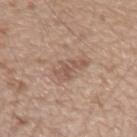Part of a total-body skin-imaging series; this lesion was reviewed on a skin check and was not flagged for biopsy. A close-up tile cropped from a whole-body skin photograph, about 15 mm across. The lesion is on the left upper arm. A male patient, about 20 years old. The lesion-visualizer software estimated a lesion area of about 11 mm², an outline eccentricity of about 0.8 (0 = round, 1 = elongated), and a symmetry-axis asymmetry near 0.25. The software also gave border irregularity of about 3 on a 0–10 scale, a within-lesion color-variation index near 3.5/10, and a peripheral color-asymmetry measure near 1.5. The analysis additionally found a nevus-likeness score of about 0/100 and a lesion-detection confidence of about 100/100.